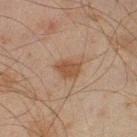The lesion was tiled from a total-body skin photograph and was not biopsied. The tile uses cross-polarized illumination. The lesion-visualizer software estimated a footprint of about 5 mm² and a shape-asymmetry score of about 0.2 (0 = symmetric). And it measured a within-lesion color-variation index near 2/10 and a peripheral color-asymmetry measure near 0.5. A male subject, in their mid- to late 40s. Approximately 3 mm at its widest. A 15 mm crop from a total-body photograph taken for skin-cancer surveillance. On the left lower leg.